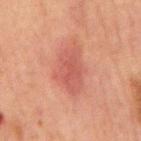follow-up: imaged on a skin check; not biopsied | TBP lesion metrics: an area of roughly 12 mm², a shape eccentricity near 0.85, and a shape-asymmetry score of about 0.3 (0 = symmetric); a lesion color around L≈44 a*≈25 b*≈24 in CIELAB and a normalized lesion–skin contrast near 6; a border-irregularity index near 3.5/10, a color-variation rating of about 2/10, and peripheral color asymmetry of about 0.5 | anatomic site: the front of the torso | imaging modality: 15 mm crop, total-body photography | lighting: cross-polarized | patient: male, aged approximately 65 | size: ≈5 mm.